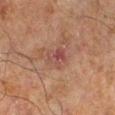Imaged during a routine full-body skin examination; the lesion was not biopsied and no histopathology is available.
The subject is a male aged around 65.
A 15 mm close-up extracted from a 3D total-body photography capture.
Located on the left lower leg.
About 2.5 mm across.
An algorithmic analysis of the crop reported a mean CIELAB color near L≈37 a*≈20 b*≈21 and a normalized border contrast of about 6.5. It also reported a within-lesion color-variation index near 4.5/10 and peripheral color asymmetry of about 1.5. And it measured a nevus-likeness score of about 0/100 and a detector confidence of about 100 out of 100 that the crop contains a lesion.
This is a cross-polarized tile.